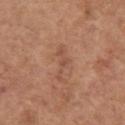Notes:
• follow-up: imaged on a skin check; not biopsied
• anatomic site: the left upper arm
• subject: female, aged 63 to 67
• image: total-body-photography crop, ~15 mm field of view
• diameter: ~4 mm (longest diameter)
• lighting: white-light